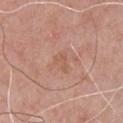The lesion was tiled from a total-body skin photograph and was not biopsied.
The lesion is located on the chest.
A 15 mm close-up tile from a total-body photography series done for melanoma screening.
A male patient, aged 58–62.